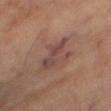Captured during whole-body skin photography for melanoma surveillance; the lesion was not biopsied. On the right thigh. Captured under cross-polarized illumination. The lesion-visualizer software estimated a footprint of about 10 mm², a shape eccentricity near 0.85, and a shape-asymmetry score of about 0.5 (0 = symmetric). It also reported a mean CIELAB color near L≈44 a*≈19 b*≈22 and about 8 CIELAB-L* units darker than the surrounding skin. And it measured a border-irregularity index near 6/10, a color-variation rating of about 4.5/10, and peripheral color asymmetry of about 1.5. A roughly 15 mm field-of-view crop from a total-body skin photograph. A subject in their mid-60s.